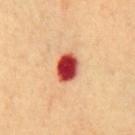Q: Was this lesion biopsied?
A: no biopsy performed (imaged during a skin exam)
Q: How was the tile lit?
A: cross-polarized illumination
Q: What did automated image analysis measure?
A: a lesion-to-skin contrast of about 15 (normalized; higher = more distinct); a border-irregularity index near 1/10, internal color variation of about 7 on a 0–10 scale, and a peripheral color-asymmetry measure near 1.5; lesion-presence confidence of about 100/100
Q: What kind of image is this?
A: ~15 mm crop, total-body skin-cancer survey
Q: Patient demographics?
A: male, in their 70s
Q: Lesion location?
A: the chest